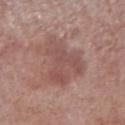workup = catalogued during a skin exam; not biopsied | anatomic site = the right lower leg | diameter = ~6 mm (longest diameter) | image = ~15 mm crop, total-body skin-cancer survey | illumination = white-light | subject = male, aged around 70.